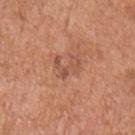  biopsy_status: not biopsied; imaged during a skin examination
  image:
    source: total-body photography crop
    field_of_view_mm: 15
  site: right upper arm
  patient:
    sex: male
    age_approx: 65
  lighting: white-light
  lesion_size:
    long_diameter_mm_approx: 3.0